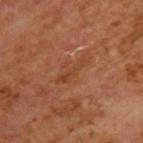Imaged during a routine full-body skin examination; the lesion was not biopsied and no histopathology is available.
The lesion-visualizer software estimated a lesion area of about 5 mm², a shape eccentricity near 0.95, and a shape-asymmetry score of about 0.4 (0 = symmetric).
Imaged with cross-polarized lighting.
A male subject, in their mid-60s.
Located on the upper back.
Cropped from a total-body skin-imaging series; the visible field is about 15 mm.
The lesion's longest dimension is about 4.5 mm.